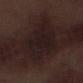Clinical impression:
Imaged during a routine full-body skin examination; the lesion was not biopsied and no histopathology is available.
Context:
On the left lower leg. A 15 mm close-up tile from a total-body photography series done for melanoma screening. Imaged with white-light lighting. The total-body-photography lesion software estimated a border-irregularity rating of about 4/10, a within-lesion color-variation index near 2.5/10, and a peripheral color-asymmetry measure near 1. It also reported a nevus-likeness score of about 0/100 and lesion-presence confidence of about 65/100. The recorded lesion diameter is about 7.5 mm. A male subject approximately 70 years of age.